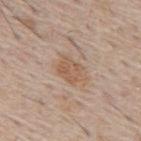Q: Is there a histopathology result?
A: catalogued during a skin exam; not biopsied
Q: What lighting was used for the tile?
A: white-light illumination
Q: Lesion size?
A: about 3.5 mm
Q: What kind of image is this?
A: 15 mm crop, total-body photography
Q: Lesion location?
A: the mid back
Q: Patient demographics?
A: male, roughly 55 years of age
Q: Automated lesion metrics?
A: a footprint of about 7 mm², an eccentricity of roughly 0.7, and two-axis asymmetry of about 0.25